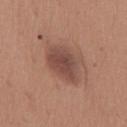follow-up: total-body-photography surveillance lesion; no biopsy | site: the chest | image-analysis metrics: a shape eccentricity near 0.7 and two-axis asymmetry of about 0.2; an average lesion color of about L≈46 a*≈21 b*≈25 (CIELAB), roughly 11 lightness units darker than nearby skin, and a normalized border contrast of about 8; a border-irregularity index near 2/10 and internal color variation of about 3 on a 0–10 scale | subject: female, aged around 65 | acquisition: total-body-photography crop, ~15 mm field of view | size: ~5 mm (longest diameter).